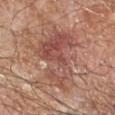notes: total-body-photography surveillance lesion; no biopsy
anatomic site: the right forearm
lesion size: ≈7.5 mm
image source: ~15 mm crop, total-body skin-cancer survey
illumination: cross-polarized illumination
subject: male, about 65 years old